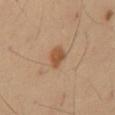This lesion was catalogued during total-body skin photography and was not selected for biopsy. About 3 mm across. Located on the mid back. A 15 mm close-up extracted from a 3D total-body photography capture. This is a cross-polarized tile. A male patient about 40 years old.